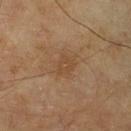<tbp_lesion>
<biopsy_status>not biopsied; imaged during a skin examination</biopsy_status>
<automated_metrics>
  <shape_asymmetry>0.35</shape_asymmetry>
  <color_variation_0_10>1.5</color_variation_0_10>
  <peripheral_color_asymmetry>0.5</peripheral_color_asymmetry>
  <nevus_likeness_0_100>0</nevus_likeness_0_100>
  <lesion_detection_confidence_0_100>100</lesion_detection_confidence_0_100>
</automated_metrics>
<lighting>cross-polarized</lighting>
<patient>
  <sex>male</sex>
  <age_approx>65</age_approx>
</patient>
<site>right lower leg</site>
<lesion_size>
  <long_diameter_mm_approx>2.5</long_diameter_mm_approx>
</lesion_size>
<image>
  <source>total-body photography crop</source>
  <field_of_view_mm>15</field_of_view_mm>
</image>
</tbp_lesion>